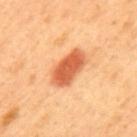Assessment:
Recorded during total-body skin imaging; not selected for excision or biopsy.
Acquisition and patient details:
The patient is a male aged around 50. On the back. This image is a 15 mm lesion crop taken from a total-body photograph.